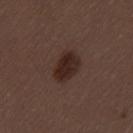The subject is a female in their 50s.
The recorded lesion diameter is about 4 mm.
This image is a 15 mm lesion crop taken from a total-body photograph.
On the left thigh.